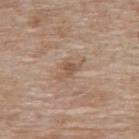site: upper back
lesion_size:
  long_diameter_mm_approx: 3.5
image:
  source: total-body photography crop
  field_of_view_mm: 15
patient:
  sex: female
  age_approx: 75
lighting: white-light